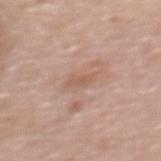Notes:
- notes — no biopsy performed (imaged during a skin exam)
- image-analysis metrics — an area of roughly 2.5 mm², an eccentricity of roughly 0.9, and a symmetry-axis asymmetry near 0.45; a mean CIELAB color near L≈57 a*≈20 b*≈28 and a normalized lesion–skin contrast near 5.5; peripheral color asymmetry of about 0
- image — 15 mm crop, total-body photography
- patient — female, about 60 years old
- lesion size — about 3 mm
- body site — the upper back
- lighting — white-light